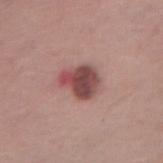This lesion was catalogued during total-body skin photography and was not selected for biopsy.
Approximately 4 mm at its widest.
A 15 mm close-up tile from a total-body photography series done for melanoma screening.
The lesion-visualizer software estimated a lesion color around L≈47 a*≈25 b*≈21 in CIELAB, about 15 CIELAB-L* units darker than the surrounding skin, and a lesion-to-skin contrast of about 10.5 (normalized; higher = more distinct). The software also gave a border-irregularity index near 3/10, internal color variation of about 5.5 on a 0–10 scale, and a peripheral color-asymmetry measure near 1.5. The software also gave a nevus-likeness score of about 80/100 and a detector confidence of about 100 out of 100 that the crop contains a lesion.
A female patient aged around 40.
Captured under white-light illumination.
The lesion is located on the abdomen.